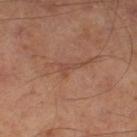Recorded during total-body skin imaging; not selected for excision or biopsy. This image is a 15 mm lesion crop taken from a total-body photograph. The tile uses cross-polarized illumination. A male subject, aged around 65.